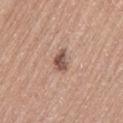<case>
<biopsy_status>not biopsied; imaged during a skin examination</biopsy_status>
<automated_metrics>
  <area_mm2_approx>4.5</area_mm2_approx>
  <eccentricity>0.8</eccentricity>
  <shape_asymmetry>0.35</shape_asymmetry>
  <cielab_L>53</cielab_L>
  <cielab_a>19</cielab_a>
  <cielab_b>26</cielab_b>
  <vs_skin_darker_L>13.0</vs_skin_darker_L>
  <border_irregularity_0_10>3.5</border_irregularity_0_10>
  <color_variation_0_10>4.0</color_variation_0_10>
  <peripheral_color_asymmetry>1.5</peripheral_color_asymmetry>
  <nevus_likeness_0_100>80</nevus_likeness_0_100>
  <lesion_detection_confidence_0_100>100</lesion_detection_confidence_0_100>
</automated_metrics>
<lighting>white-light</lighting>
<patient>
  <sex>female</sex>
  <age_approx>50</age_approx>
</patient>
<image>
  <source>total-body photography crop</source>
  <field_of_view_mm>15</field_of_view_mm>
</image>
<site>left thigh</site>
</case>